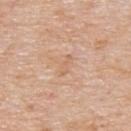Captured during whole-body skin photography for melanoma surveillance; the lesion was not biopsied.
From the upper back.
The patient is a male in their mid-70s.
The recorded lesion diameter is about 2.5 mm.
A 15 mm close-up tile from a total-body photography series done for melanoma screening.
The total-body-photography lesion software estimated a lesion area of about 2 mm² and an eccentricity of roughly 0.95. The analysis additionally found an automated nevus-likeness rating near 0 out of 100.